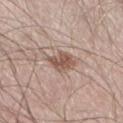Context: Located on the leg. A 15 mm close-up tile from a total-body photography series done for melanoma screening. A male subject, aged 53–57. Captured under white-light illumination. The recorded lesion diameter is about 3 mm.